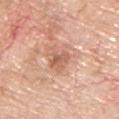The lesion was photographed on a routine skin check and not biopsied; there is no pathology result.
Imaged with white-light lighting.
The lesion is on the upper back.
A male patient, aged 63–67.
The lesion's longest dimension is about 3 mm.
A 15 mm close-up extracted from a 3D total-body photography capture.
An algorithmic analysis of the crop reported roughly 10 lightness units darker than nearby skin and a normalized lesion–skin contrast near 6.